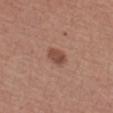<case>
  <biopsy_status>not biopsied; imaged during a skin examination</biopsy_status>
  <site>arm</site>
  <image>
    <source>total-body photography crop</source>
    <field_of_view_mm>15</field_of_view_mm>
  </image>
  <patient>
    <sex>male</sex>
    <age_approx>75</age_approx>
  </patient>
  <automated_metrics>
    <cielab_L>47</cielab_L>
    <cielab_a>22</cielab_a>
    <cielab_b>27</cielab_b>
    <vs_skin_darker_L>11.0</vs_skin_darker_L>
    <vs_skin_contrast_norm>8.0</vs_skin_contrast_norm>
    <border_irregularity_0_10>1.5</border_irregularity_0_10>
    <color_variation_0_10>2.5</color_variation_0_10>
    <peripheral_color_asymmetry>1.0</peripheral_color_asymmetry>
  </automated_metrics>
  <lighting>white-light</lighting>
</case>